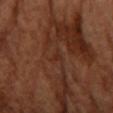| key | value |
|---|---|
| follow-up | catalogued during a skin exam; not biopsied |
| TBP lesion metrics | a footprint of about 1 mm², a shape eccentricity near 0.7, and two-axis asymmetry of about 0.4; a lesion color around L≈27 a*≈22 b*≈27 in CIELAB, roughly 4 lightness units darker than nearby skin, and a normalized lesion–skin contrast near 5; a nevus-likeness score of about 0/100 and a detector confidence of about 95 out of 100 that the crop contains a lesion |
| image | ~15 mm tile from a whole-body skin photo |
| lesion size | ≈1 mm |
| anatomic site | the right forearm |
| patient | female, aged approximately 65 |
| illumination | cross-polarized illumination |